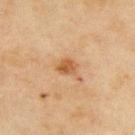The lesion was photographed on a routine skin check and not biopsied; there is no pathology result. A region of skin cropped from a whole-body photographic capture, roughly 15 mm wide. The lesion is located on the upper back. The patient is a female in their 60s.